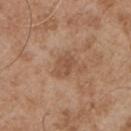Captured during whole-body skin photography for melanoma surveillance; the lesion was not biopsied. A male patient, in their mid-50s. The lesion is on the chest. A region of skin cropped from a whole-body photographic capture, roughly 15 mm wide.